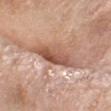Part of a total-body skin-imaging series; this lesion was reviewed on a skin check and was not flagged for biopsy. A female subject, roughly 75 years of age. A 15 mm close-up tile from a total-body photography series done for melanoma screening. The lesion is on the left forearm. Captured under white-light illumination. The total-body-photography lesion software estimated an average lesion color of about L≈55 a*≈22 b*≈29 (CIELAB) and about 13 CIELAB-L* units darker than the surrounding skin. The software also gave a nevus-likeness score of about 0/100 and a detector confidence of about 55 out of 100 that the crop contains a lesion.